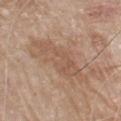biopsy status — no biopsy performed (imaged during a skin exam)
site — the front of the torso
automated lesion analysis — a lesion area of about 16 mm² and an eccentricity of roughly 0.95
lesion size — ≈8 mm
tile lighting — white-light
subject — male, aged around 80
imaging modality — total-body-photography crop, ~15 mm field of view